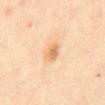biopsy status = no biopsy performed (imaged during a skin exam) | illumination = cross-polarized illumination | image = ~15 mm crop, total-body skin-cancer survey | image-analysis metrics = an area of roughly 5 mm² and a symmetry-axis asymmetry near 0.25; an automated nevus-likeness rating near 65 out of 100 and lesion-presence confidence of about 100/100 | anatomic site = the front of the torso | diameter = ≈2.5 mm | subject = male, aged 43–47.